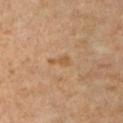<tbp_lesion>
<biopsy_status>not biopsied; imaged during a skin examination</biopsy_status>
<lighting>cross-polarized</lighting>
<patient>
  <sex>female</sex>
  <age_approx>60</age_approx>
</patient>
<image>
  <source>total-body photography crop</source>
  <field_of_view_mm>15</field_of_view_mm>
</image>
<site>chest</site>
</tbp_lesion>